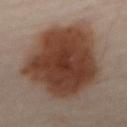{
  "biopsy_status": "not biopsied; imaged during a skin examination",
  "patient": {
    "sex": "male",
    "age_approx": 65
  },
  "site": "front of the torso",
  "image": {
    "source": "total-body photography crop",
    "field_of_view_mm": 15
  }
}